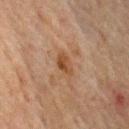notes: catalogued during a skin exam; not biopsied | diameter: ≈3 mm | illumination: cross-polarized illumination | automated lesion analysis: an average lesion color of about L≈37 a*≈18 b*≈29 (CIELAB) and a normalized lesion–skin contrast near 8 | image: ~15 mm tile from a whole-body skin photo | anatomic site: the mid back | subject: male, in their mid-60s.